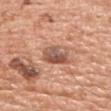This lesion was catalogued during total-body skin photography and was not selected for biopsy. The lesion is located on the head or neck. A close-up tile cropped from a whole-body skin photograph, about 15 mm across. The subject is a male aged 68 to 72.